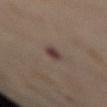The lesion was photographed on a routine skin check and not biopsied; there is no pathology result.
The lesion's longest dimension is about 2.5 mm.
On the mid back.
A 15 mm close-up extracted from a 3D total-body photography capture.
Captured under cross-polarized illumination.
The subject is a female aged around 55.